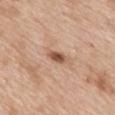This lesion was catalogued during total-body skin photography and was not selected for biopsy.
The total-body-photography lesion software estimated a lesion area of about 3.5 mm², a shape eccentricity near 0.85, and a shape-asymmetry score of about 0.35 (0 = symmetric). It also reported a border-irregularity rating of about 3/10, a color-variation rating of about 2.5/10, and a peripheral color-asymmetry measure near 0.5. It also reported a nevus-likeness score of about 90/100 and a lesion-detection confidence of about 100/100.
The subject is a female about 40 years old.
Measured at roughly 2.5 mm in maximum diameter.
Cropped from a whole-body photographic skin survey; the tile spans about 15 mm.
The lesion is located on the mid back.